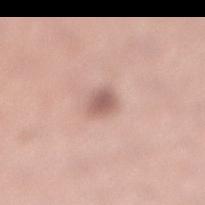Q: Was a biopsy performed?
A: total-body-photography surveillance lesion; no biopsy